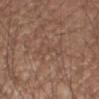Q: Was a biopsy performed?
A: catalogued during a skin exam; not biopsied
Q: Lesion size?
A: ≈5 mm
Q: Where on the body is the lesion?
A: the right upper arm
Q: Automated lesion metrics?
A: an outline eccentricity of about 0.95 (0 = round, 1 = elongated) and a shape-asymmetry score of about 0.4 (0 = symmetric)
Q: What is the imaging modality?
A: 15 mm crop, total-body photography
Q: Illumination type?
A: white-light illumination
Q: Patient demographics?
A: male, about 55 years old